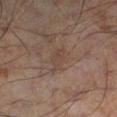notes — catalogued during a skin exam; not biopsied | patient — male, approximately 55 years of age | illumination — cross-polarized illumination | image-analysis metrics — an outline eccentricity of about 0.85 (0 = round, 1 = elongated); a mean CIELAB color near L≈40 a*≈14 b*≈21 and a normalized lesion–skin contrast near 5; a border-irregularity rating of about 3.5/10 and a color-variation rating of about 2.5/10 | image source — ~15 mm tile from a whole-body skin photo | site — the left lower leg.